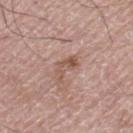Acquisition and patient details: A region of skin cropped from a whole-body photographic capture, roughly 15 mm wide. An algorithmic analysis of the crop reported an area of roughly 4.5 mm² and a symmetry-axis asymmetry near 0.55. The software also gave an average lesion color of about L≈54 a*≈20 b*≈26 (CIELAB), about 9 CIELAB-L* units darker than the surrounding skin, and a normalized border contrast of about 7. The analysis additionally found a color-variation rating of about 1.5/10 and radial color variation of about 0.5. Captured under white-light illumination. A male patient in their mid- to late 70s. The lesion is on the left thigh.